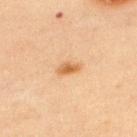Captured during whole-body skin photography for melanoma surveillance; the lesion was not biopsied. A region of skin cropped from a whole-body photographic capture, roughly 15 mm wide. Longest diameter approximately 3 mm. Automated tile analysis of the lesion measured a footprint of about 3.5 mm² and an eccentricity of roughly 0.85. It also reported an average lesion color of about L≈53 a*≈20 b*≈36 (CIELAB) and a normalized border contrast of about 8. The analysis additionally found a nevus-likeness score of about 90/100. The patient is a male approximately 60 years of age. From the upper back. The tile uses cross-polarized illumination.